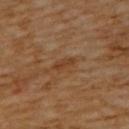Q: Was a biopsy performed?
A: total-body-photography surveillance lesion; no biopsy
Q: How was the tile lit?
A: cross-polarized
Q: What are the patient's age and sex?
A: female, aged around 65
Q: Lesion location?
A: the back
Q: What is the lesion's diameter?
A: about 3 mm
Q: How was this image acquired?
A: total-body-photography crop, ~15 mm field of view